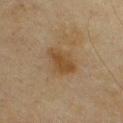{"biopsy_status": "not biopsied; imaged during a skin examination", "site": "chest", "patient": {"sex": "male", "age_approx": 65}, "image": {"source": "total-body photography crop", "field_of_view_mm": 15}, "lesion_size": {"long_diameter_mm_approx": 4.0}}